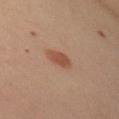Automated image analysis of the tile measured a lesion area of about 4.5 mm², an outline eccentricity of about 0.8 (0 = round, 1 = elongated), and a symmetry-axis asymmetry near 0.2. The software also gave about 10 CIELAB-L* units darker than the surrounding skin and a normalized border contrast of about 7.5. The software also gave a border-irregularity index near 2/10, internal color variation of about 1.5 on a 0–10 scale, and peripheral color asymmetry of about 0.5. The software also gave an automated nevus-likeness rating near 100 out of 100 and a detector confidence of about 100 out of 100 that the crop contains a lesion. Approximately 3 mm at its widest. Cropped from a total-body skin-imaging series; the visible field is about 15 mm. This is a cross-polarized tile. Located on the right upper arm. A female subject, aged 38–42.